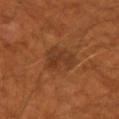{
  "biopsy_status": "not biopsied; imaged during a skin examination",
  "automated_metrics": {
    "area_mm2_approx": 10.0
  },
  "site": "left upper arm",
  "lighting": "cross-polarized",
  "patient": {
    "sex": "male",
    "age_approx": 50
  },
  "image": {
    "source": "total-body photography crop",
    "field_of_view_mm": 15
  },
  "lesion_size": {
    "long_diameter_mm_approx": 4.0
  }
}